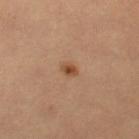| key | value |
|---|---|
| biopsy status | imaged on a skin check; not biopsied |
| acquisition | ~15 mm tile from a whole-body skin photo |
| illumination | cross-polarized |
| subject | female, approximately 30 years of age |
| anatomic site | the left thigh |